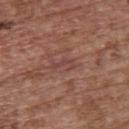<record>
  <biopsy_status>not biopsied; imaged during a skin examination</biopsy_status>
  <lighting>white-light</lighting>
  <lesion_size>
    <long_diameter_mm_approx>3.0</long_diameter_mm_approx>
  </lesion_size>
  <automated_metrics>
    <border_irregularity_0_10>7.0</border_irregularity_0_10>
    <color_variation_0_10>0.0</color_variation_0_10>
    <peripheral_color_asymmetry>0.0</peripheral_color_asymmetry>
    <nevus_likeness_0_100>0</nevus_likeness_0_100>
    <lesion_detection_confidence_0_100>50</lesion_detection_confidence_0_100>
  </automated_metrics>
  <site>upper back</site>
  <image>
    <source>total-body photography crop</source>
    <field_of_view_mm>15</field_of_view_mm>
  </image>
  <patient>
    <sex>female</sex>
    <age_approx>65</age_approx>
  </patient>
</record>